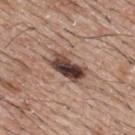notes: total-body-photography surveillance lesion; no biopsy
site: the upper back
image source: 15 mm crop, total-body photography
TBP lesion metrics: a lesion area of about 10 mm² and a symmetry-axis asymmetry near 0.2; radial color variation of about 4; a nevus-likeness score of about 100/100 and a lesion-detection confidence of about 100/100
patient: male, roughly 65 years of age